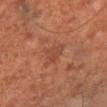No biopsy was performed on this lesion — it was imaged during a full skin examination and was not determined to be concerning. The lesion is on the right lower leg. Cropped from a whole-body photographic skin survey; the tile spans about 15 mm. A male subject, roughly 70 years of age.